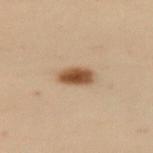* notes · no biopsy performed (imaged during a skin exam)
* subject · female, approximately 50 years of age
* anatomic site · the left upper arm
* diameter · about 3 mm
* image · 15 mm crop, total-body photography